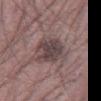Assessment: Part of a total-body skin-imaging series; this lesion was reviewed on a skin check and was not flagged for biopsy. Context: Automated tile analysis of the lesion measured an area of roughly 12 mm², an eccentricity of roughly 0.85, and a symmetry-axis asymmetry near 0.2. It also reported a lesion–skin lightness drop of about 11 and a lesion-to-skin contrast of about 8.5 (normalized; higher = more distinct). And it measured a border-irregularity rating of about 3/10, internal color variation of about 3.5 on a 0–10 scale, and a peripheral color-asymmetry measure near 1. The recorded lesion diameter is about 5 mm. The subject is a male aged around 60. This is a white-light tile. A close-up tile cropped from a whole-body skin photograph, about 15 mm across. From the right thigh.